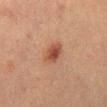Q: Was this lesion biopsied?
A: no biopsy performed (imaged during a skin exam)
Q: Who is the patient?
A: female, roughly 55 years of age
Q: Lesion location?
A: the right lower leg
Q: How was this image acquired?
A: ~15 mm tile from a whole-body skin photo